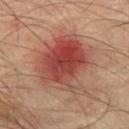follow-up: catalogued during a skin exam; not biopsied | imaging modality: ~15 mm tile from a whole-body skin photo | automated lesion analysis: a lesion area of about 34 mm², an outline eccentricity of about 0.45 (0 = round, 1 = elongated), and two-axis asymmetry of about 0.25; a mean CIELAB color near L≈39 a*≈24 b*≈24, about 10 CIELAB-L* units darker than the surrounding skin, and a normalized lesion–skin contrast near 8.5; a border-irregularity index near 3.5/10 and a color-variation rating of about 9/10 | location: the left thigh | lighting: cross-polarized illumination | patient: male, approximately 75 years of age | diameter: about 7 mm.